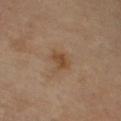Assessment:
The lesion was tiled from a total-body skin photograph and was not biopsied.
Context:
Cropped from a whole-body photographic skin survey; the tile spans about 15 mm. The lesion is on the right upper arm. A male subject, aged approximately 70.